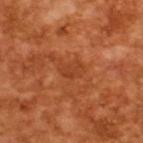| feature | finding |
|---|---|
| biopsy status | imaged on a skin check; not biopsied |
| automated lesion analysis | an eccentricity of roughly 0.8 and a symmetry-axis asymmetry near 0.35; a mean CIELAB color near L≈41 a*≈30 b*≈39; border irregularity of about 3.5 on a 0–10 scale and internal color variation of about 2 on a 0–10 scale |
| illumination | cross-polarized illumination |
| diameter | ~2.5 mm (longest diameter) |
| subject | male, aged 63 to 67 |
| image source | ~15 mm crop, total-body skin-cancer survey |